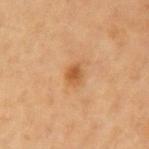notes: catalogued during a skin exam; not biopsied | size: ≈2.5 mm | image-analysis metrics: a lesion color around L≈47 a*≈21 b*≈36 in CIELAB, roughly 9 lightness units darker than nearby skin, and a lesion-to-skin contrast of about 7.5 (normalized; higher = more distinct); a within-lesion color-variation index near 2/10 and peripheral color asymmetry of about 0.5; a classifier nevus-likeness of about 80/100 and a lesion-detection confidence of about 100/100 | patient: female, aged 38–42 | image source: ~15 mm tile from a whole-body skin photo | location: the left upper arm | illumination: cross-polarized.